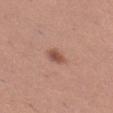Assessment:
Recorded during total-body skin imaging; not selected for excision or biopsy.
Background:
Longest diameter approximately 2.5 mm. A female patient, approximately 30 years of age. Cropped from a whole-body photographic skin survey; the tile spans about 15 mm. The tile uses white-light illumination. The lesion is on the left thigh. Automated image analysis of the tile measured a footprint of about 3.5 mm² and a shape-asymmetry score of about 0.25 (0 = symmetric). And it measured border irregularity of about 2 on a 0–10 scale, internal color variation of about 3 on a 0–10 scale, and peripheral color asymmetry of about 1. The software also gave a lesion-detection confidence of about 100/100.